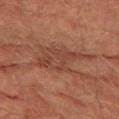Clinical impression:
The lesion was tiled from a total-body skin photograph and was not biopsied.
Clinical summary:
On the leg. The total-body-photography lesion software estimated a footprint of about 16 mm² and a shape-asymmetry score of about 0.4 (0 = symmetric). It also reported a border-irregularity index near 6.5/10, a color-variation rating of about 3/10, and a peripheral color-asymmetry measure near 1. The software also gave a nevus-likeness score of about 0/100 and a lesion-detection confidence of about 80/100. This image is a 15 mm lesion crop taken from a total-body photograph. Measured at roughly 6 mm in maximum diameter. The tile uses cross-polarized illumination. The patient is a male roughly 75 years of age.